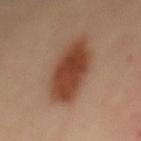This lesion was catalogued during total-body skin photography and was not selected for biopsy.
This image is a 15 mm lesion crop taken from a total-body photograph.
The lesion is on the back.
This is a cross-polarized tile.
A female patient, aged approximately 35.